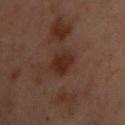<lesion>
<biopsy_status>not biopsied; imaged during a skin examination</biopsy_status>
<site>left upper arm</site>
<patient>
  <sex>female</sex>
  <age_approx>55</age_approx>
</patient>
<image>
  <source>total-body photography crop</source>
  <field_of_view_mm>15</field_of_view_mm>
</image>
</lesion>